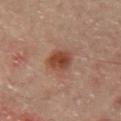A roughly 15 mm field-of-view crop from a total-body skin photograph. A male patient, aged around 65. Located on the mid back.